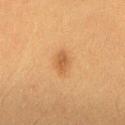<lesion>
<biopsy_status>not biopsied; imaged during a skin examination</biopsy_status>
<patient>
  <sex>female</sex>
  <age_approx>40</age_approx>
</patient>
<lesion_size>
  <long_diameter_mm_approx>3.0</long_diameter_mm_approx>
</lesion_size>
<site>right thigh</site>
<image>
  <source>total-body photography crop</source>
  <field_of_view_mm>15</field_of_view_mm>
</image>
<lighting>cross-polarized</lighting>
</lesion>